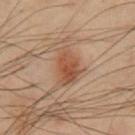Captured during whole-body skin photography for melanoma surveillance; the lesion was not biopsied.
The recorded lesion diameter is about 4 mm.
Automated tile analysis of the lesion measured an eccentricity of roughly 0.75. And it measured a lesion–skin lightness drop of about 8 and a normalized border contrast of about 7.5. And it measured a nevus-likeness score of about 90/100.
Located on the chest.
The patient is a male in their mid- to late 50s.
A close-up tile cropped from a whole-body skin photograph, about 15 mm across.